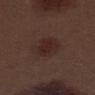Assessment: This lesion was catalogued during total-body skin photography and was not selected for biopsy. Image and clinical context: On the left thigh. The total-body-photography lesion software estimated an area of roughly 9 mm², an eccentricity of roughly 0.45, and a shape-asymmetry score of about 0.15 (0 = symmetric). The software also gave a nevus-likeness score of about 75/100 and lesion-presence confidence of about 100/100. Imaged with white-light lighting. This image is a 15 mm lesion crop taken from a total-body photograph. A male patient, approximately 70 years of age.